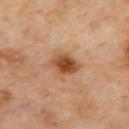Impression: The lesion was photographed on a routine skin check and not biopsied; there is no pathology result. Background: From the mid back. Measured at roughly 3.5 mm in maximum diameter. Cropped from a whole-body photographic skin survey; the tile spans about 15 mm. Automated image analysis of the tile measured a lesion color around L≈52 a*≈23 b*≈37 in CIELAB and a lesion–skin lightness drop of about 13. The analysis additionally found a nevus-likeness score of about 95/100 and a detector confidence of about 100 out of 100 that the crop contains a lesion. Imaged with cross-polarized lighting.